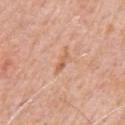Background: A male subject aged approximately 80. The lesion is on the chest. This image is a 15 mm lesion crop taken from a total-body photograph.